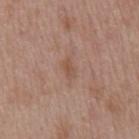Q: What kind of image is this?
A: ~15 mm tile from a whole-body skin photo
Q: Who is the patient?
A: male, approximately 55 years of age
Q: What is the lesion's diameter?
A: ≈2.5 mm
Q: Illumination type?
A: white-light illumination
Q: What did automated image analysis measure?
A: an eccentricity of roughly 0.85 and two-axis asymmetry of about 0.3; an average lesion color of about L≈52 a*≈19 b*≈28 (CIELAB), a lesion–skin lightness drop of about 6, and a normalized border contrast of about 5.5; a border-irregularity index near 3/10, a within-lesion color-variation index near 1/10, and radial color variation of about 0
Q: Where on the body is the lesion?
A: the abdomen